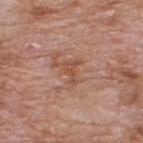• notes · catalogued during a skin exam; not biopsied
• site · the upper back
• tile lighting · white-light
• image · ~15 mm tile from a whole-body skin photo
• image-analysis metrics · a footprint of about 4 mm² and an eccentricity of roughly 0.8; a lesion color around L≈51 a*≈23 b*≈31 in CIELAB and a lesion–skin lightness drop of about 7; a border-irregularity rating of about 6/10, a within-lesion color-variation index near 1.5/10, and a peripheral color-asymmetry measure near 0.5
• patient · male, aged around 60
• size · about 3 mm